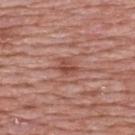Q: What kind of image is this?
A: 15 mm crop, total-body photography
Q: How was the tile lit?
A: white-light
Q: What are the patient's age and sex?
A: male, aged approximately 50
Q: Where on the body is the lesion?
A: the upper back